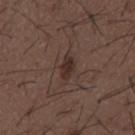  image:
    source: total-body photography crop
    field_of_view_mm: 15
  patient:
    sex: male
    age_approx: 50
  site: mid back